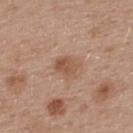Assessment: Captured during whole-body skin photography for melanoma surveillance; the lesion was not biopsied. Clinical summary: About 3 mm across. Located on the upper back. Automated tile analysis of the lesion measured an area of roughly 5.5 mm² and a shape-asymmetry score of about 0.2 (0 = symmetric). It also reported a lesion color around L≈53 a*≈20 b*≈30 in CIELAB, about 9 CIELAB-L* units darker than the surrounding skin, and a normalized border contrast of about 6.5. The analysis additionally found a border-irregularity index near 2/10, a within-lesion color-variation index near 3.5/10, and peripheral color asymmetry of about 1. The software also gave an automated nevus-likeness rating near 20 out of 100 and a lesion-detection confidence of about 100/100. This is a white-light tile. A female subject about 40 years old. This image is a 15 mm lesion crop taken from a total-body photograph.